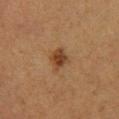Q: Is there a histopathology result?
A: total-body-photography surveillance lesion; no biopsy